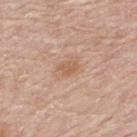Imaged during a routine full-body skin examination; the lesion was not biopsied and no histopathology is available. A male subject approximately 80 years of age. Captured under white-light illumination. Cropped from a total-body skin-imaging series; the visible field is about 15 mm. The lesion is located on the back. The recorded lesion diameter is about 2.5 mm. Automated tile analysis of the lesion measured an eccentricity of roughly 0.8.